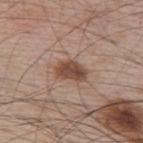Assessment: Imaged during a routine full-body skin examination; the lesion was not biopsied and no histopathology is available. Context: Automated tile analysis of the lesion measured a lesion-to-skin contrast of about 9.5 (normalized; higher = more distinct). From the upper back. Captured under white-light illumination. A male subject, aged approximately 65. A 15 mm close-up extracted from a 3D total-body photography capture.